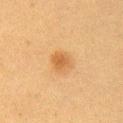Findings:
- site — the arm
- image — ~15 mm tile from a whole-body skin photo
- patient — female, in their 40s
- size — ~2.5 mm (longest diameter)
- illumination — cross-polarized illumination